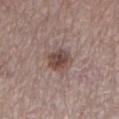Q: Is there a histopathology result?
A: total-body-photography surveillance lesion; no biopsy
Q: Who is the patient?
A: female, in their mid- to late 60s
Q: What kind of image is this?
A: ~15 mm crop, total-body skin-cancer survey
Q: How was the tile lit?
A: white-light
Q: What is the anatomic site?
A: the left lower leg